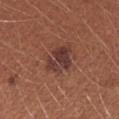Recorded during total-body skin imaging; not selected for excision or biopsy.
Cropped from a total-body skin-imaging series; the visible field is about 15 mm.
Imaged with white-light lighting.
A female patient, about 25 years old.
On the arm.
Approximately 4.5 mm at its widest.